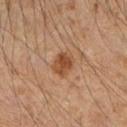workup: imaged on a skin check; not biopsied
image: total-body-photography crop, ~15 mm field of view
patient: male, aged approximately 50
lesion diameter: about 3 mm
body site: the right upper arm